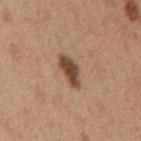Q: Is there a histopathology result?
A: total-body-photography surveillance lesion; no biopsy
Q: How was this image acquired?
A: ~15 mm tile from a whole-body skin photo
Q: How was the tile lit?
A: white-light
Q: How large is the lesion?
A: ~4.5 mm (longest diameter)
Q: Who is the patient?
A: female, aged approximately 40
Q: What did automated image analysis measure?
A: a mean CIELAB color near L≈46 a*≈21 b*≈28, about 16 CIELAB-L* units darker than the surrounding skin, and a normalized border contrast of about 11; a border-irregularity rating of about 3/10, a within-lesion color-variation index near 4/10, and radial color variation of about 1.5; a detector confidence of about 100 out of 100 that the crop contains a lesion
Q: Where on the body is the lesion?
A: the mid back